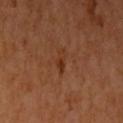A close-up tile cropped from a whole-body skin photograph, about 15 mm across.
The subject is a female in their 40s.
From the left upper arm.
This is a cross-polarized tile.